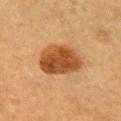Clinical impression:
Part of a total-body skin-imaging series; this lesion was reviewed on a skin check and was not flagged for biopsy.
Clinical summary:
A region of skin cropped from a whole-body photographic capture, roughly 15 mm wide. Captured under cross-polarized illumination. A female subject, aged around 55. The lesion is located on the arm. Longest diameter approximately 5.5 mm.